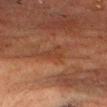This lesion was catalogued during total-body skin photography and was not selected for biopsy.
A male subject aged approximately 50.
A lesion tile, about 15 mm wide, cut from a 3D total-body photograph.
From the head or neck.